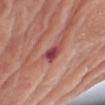This is a white-light tile. On the right upper arm. Measured at roughly 3.5 mm in maximum diameter. Automated tile analysis of the lesion measured a footprint of about 5 mm² and two-axis asymmetry of about 0.35. It also reported a lesion color around L≈47 a*≈31 b*≈23 in CIELAB, about 13 CIELAB-L* units darker than the surrounding skin, and a lesion-to-skin contrast of about 9.5 (normalized; higher = more distinct). It also reported a border-irregularity rating of about 3.5/10, a within-lesion color-variation index near 5/10, and a peripheral color-asymmetry measure near 1.5. A 15 mm close-up tile from a total-body photography series done for melanoma screening. A male patient aged 78–82.